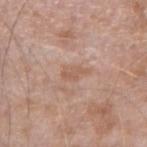Impression: Recorded during total-body skin imaging; not selected for excision or biopsy. Acquisition and patient details: The lesion is located on the right forearm. Automated image analysis of the tile measured an area of roughly 3.5 mm², an eccentricity of roughly 0.8, and a shape-asymmetry score of about 0.35 (0 = symmetric). It also reported a mean CIELAB color near L≈58 a*≈19 b*≈29 and about 7 CIELAB-L* units darker than the surrounding skin. The software also gave a border-irregularity rating of about 4/10, a within-lesion color-variation index near 1/10, and radial color variation of about 0.5. A close-up tile cropped from a whole-body skin photograph, about 15 mm across. Imaged with white-light lighting. A male patient aged around 75.